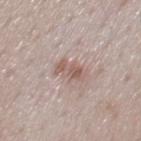Q: Is there a histopathology result?
A: no biopsy performed (imaged during a skin exam)
Q: What are the patient's age and sex?
A: male, aged approximately 50
Q: How large is the lesion?
A: ~3 mm (longest diameter)
Q: Where on the body is the lesion?
A: the mid back
Q: What did automated image analysis measure?
A: an area of roughly 5 mm² and a shape eccentricity near 0.85; a lesion color around L≈57 a*≈18 b*≈23 in CIELAB and a lesion-to-skin contrast of about 6.5 (normalized; higher = more distinct); border irregularity of about 4 on a 0–10 scale, a color-variation rating of about 2.5/10, and peripheral color asymmetry of about 0.5; an automated nevus-likeness rating near 0 out of 100
Q: What is the imaging modality?
A: ~15 mm tile from a whole-body skin photo